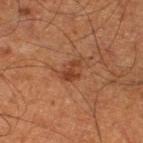<record>
<biopsy_status>not biopsied; imaged during a skin examination</biopsy_status>
<site>left lower leg</site>
<image>
  <source>total-body photography crop</source>
  <field_of_view_mm>15</field_of_view_mm>
</image>
<lighting>cross-polarized</lighting>
<patient>
  <sex>male</sex>
  <age_approx>65</age_approx>
</patient>
</record>